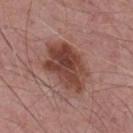Case summary:
* diameter · ~6.5 mm (longest diameter)
* illumination · white-light
* subject · male, in their mid-70s
* image source · ~15 mm crop, total-body skin-cancer survey
* location · the chest